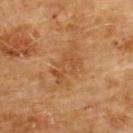| field | value |
|---|---|
| workup | imaged on a skin check; not biopsied |
| imaging modality | 15 mm crop, total-body photography |
| site | the chest |
| patient | male, roughly 60 years of age |
| lighting | cross-polarized |